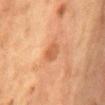<case>
  <biopsy_status>not biopsied; imaged during a skin examination</biopsy_status>
  <image>
    <source>total-body photography crop</source>
    <field_of_view_mm>15</field_of_view_mm>
  </image>
  <automated_metrics>
    <area_mm2_approx>4.0</area_mm2_approx>
    <eccentricity>0.7</eccentricity>
    <shape_asymmetry>0.2</shape_asymmetry>
    <cielab_L>48</cielab_L>
    <cielab_a>22</cielab_a>
    <cielab_b>33</cielab_b>
    <vs_skin_darker_L>8.0</vs_skin_darker_L>
    <vs_skin_contrast_norm>6.0</vs_skin_contrast_norm>
    <border_irregularity_0_10>2.0</border_irregularity_0_10>
  </automated_metrics>
  <site>chest</site>
  <lesion_size>
    <long_diameter_mm_approx>2.5</long_diameter_mm_approx>
  </lesion_size>
  <patient>
    <sex>female</sex>
    <age_approx>50</age_approx>
  </patient>
  <lighting>cross-polarized</lighting>
</case>